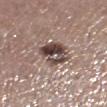{"biopsy_status": "not biopsied; imaged during a skin examination", "patient": {"sex": "female", "age_approx": 65}, "image": {"source": "total-body photography crop", "field_of_view_mm": 15}, "lighting": "white-light", "site": "left lower leg", "lesion_size": {"long_diameter_mm_approx": 4.0}}